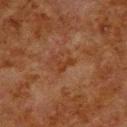- notes: no biopsy performed (imaged during a skin exam)
- subject: male, in their 80s
- image source: ~15 mm crop, total-body skin-cancer survey
- body site: the upper back